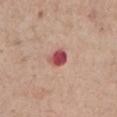No biopsy was performed on this lesion — it was imaged during a full skin examination and was not determined to be concerning.
A 15 mm crop from a total-body photograph taken for skin-cancer surveillance.
The subject is a male in their mid-70s.
The lesion is located on the chest.
Captured under white-light illumination.